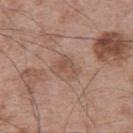Notes:
– patient — male, roughly 65 years of age
– image-analysis metrics — a border-irregularity index near 4.5/10, a within-lesion color-variation index near 3.5/10, and radial color variation of about 1; an automated nevus-likeness rating near 0 out of 100 and a detector confidence of about 100 out of 100 that the crop contains a lesion
– imaging modality — total-body-photography crop, ~15 mm field of view
– anatomic site — the upper back
– tile lighting — white-light